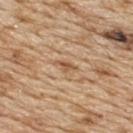Part of a total-body skin-imaging series; this lesion was reviewed on a skin check and was not flagged for biopsy. A roughly 15 mm field-of-view crop from a total-body skin photograph. A male patient, aged 68 to 72. Automated image analysis of the tile measured a lesion color around L≈55 a*≈20 b*≈35 in CIELAB, a lesion–skin lightness drop of about 10, and a normalized border contrast of about 7. Measured at roughly 2.5 mm in maximum diameter. From the upper back.